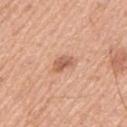Q: Was this lesion biopsied?
A: no biopsy performed (imaged during a skin exam)
Q: Patient demographics?
A: male, about 55 years old
Q: How was the tile lit?
A: white-light
Q: Lesion location?
A: the left upper arm
Q: Automated lesion metrics?
A: an outline eccentricity of about 0.75 (0 = round, 1 = elongated) and a symmetry-axis asymmetry near 0.3; an average lesion color of about L≈60 a*≈23 b*≈33 (CIELAB) and a lesion-to-skin contrast of about 7.5 (normalized; higher = more distinct)
Q: How was this image acquired?
A: ~15 mm crop, total-body skin-cancer survey
Q: Lesion size?
A: about 3 mm